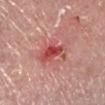  biopsy_status: not biopsied; imaged during a skin examination
  automated_metrics:
    area_mm2_approx: 7.5
    eccentricity: 0.85
    cielab_L: 51
    cielab_a: 35
    cielab_b: 28
    vs_skin_contrast_norm: 8.5
    border_irregularity_0_10: 4.5
    color_variation_0_10: 7.5
    nevus_likeness_0_100: 0
    lesion_detection_confidence_0_100: 100
  patient:
    sex: male
    age_approx: 80
  image:
    source: total-body photography crop
    field_of_view_mm: 15
  lesion_size:
    long_diameter_mm_approx: 4.0
  lighting: white-light
  site: leg